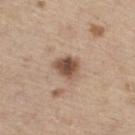From the right thigh. Approximately 3.5 mm at its widest. A region of skin cropped from a whole-body photographic capture, roughly 15 mm wide. Captured under white-light illumination. A male patient in their 70s. The lesion-visualizer software estimated border irregularity of about 2 on a 0–10 scale, a color-variation rating of about 4/10, and a peripheral color-asymmetry measure near 1.